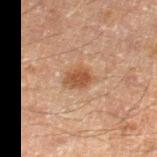illumination — cross-polarized illumination | image-analysis metrics — a footprint of about 5 mm², an eccentricity of roughly 0.65, and a symmetry-axis asymmetry near 0.2; a lesion color around L≈39 a*≈18 b*≈28 in CIELAB and about 9 CIELAB-L* units darker than the surrounding skin; border irregularity of about 2 on a 0–10 scale and internal color variation of about 2.5 on a 0–10 scale; a nevus-likeness score of about 75/100 and lesion-presence confidence of about 100/100 | image — ~15 mm tile from a whole-body skin photo | size — ~3 mm (longest diameter) | subject — male, aged around 60 | anatomic site — the right thigh.